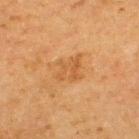Case summary:
• biopsy status — total-body-photography surveillance lesion; no biopsy
• lighting — cross-polarized
• image — 15 mm crop, total-body photography
• patient — male, approximately 65 years of age
• TBP lesion metrics — a mean CIELAB color near L≈49 a*≈21 b*≈38, about 6 CIELAB-L* units darker than the surrounding skin, and a lesion-to-skin contrast of about 5 (normalized; higher = more distinct); a border-irregularity rating of about 4.5/10; an automated nevus-likeness rating near 0 out of 100 and lesion-presence confidence of about 100/100
• anatomic site — the back